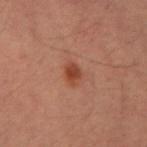{"biopsy_status": "not biopsied; imaged during a skin examination", "lesion_size": {"long_diameter_mm_approx": 3.0}, "lighting": "cross-polarized", "patient": {"sex": "male", "age_approx": 35}, "image": {"source": "total-body photography crop", "field_of_view_mm": 15}, "site": "right upper arm", "automated_metrics": {"cielab_L": 40, "cielab_a": 24, "cielab_b": 30, "vs_skin_darker_L": 9.0, "border_irregularity_0_10": 2.5, "peripheral_color_asymmetry": 1.0, "nevus_likeness_0_100": 95, "lesion_detection_confidence_0_100": 100}}